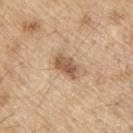Captured during whole-body skin photography for melanoma surveillance; the lesion was not biopsied.
This is a white-light tile.
A male patient aged approximately 70.
The lesion is located on the upper back.
A region of skin cropped from a whole-body photographic capture, roughly 15 mm wide.
The lesion's longest dimension is about 3.5 mm.